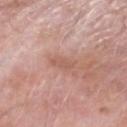{
  "image": {
    "source": "total-body photography crop",
    "field_of_view_mm": 15
  },
  "lighting": "white-light",
  "site": "left forearm",
  "patient": {
    "sex": "male",
    "age_approx": 75
  },
  "lesion_size": {
    "long_diameter_mm_approx": 3.0
  }
}